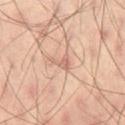| feature | finding |
|---|---|
| biopsy status | catalogued during a skin exam; not biopsied |
| body site | the right thigh |
| subject | male, aged approximately 40 |
| image | 15 mm crop, total-body photography |
| automated lesion analysis | a lesion color around L≈63 a*≈21 b*≈28 in CIELAB and a lesion-to-skin contrast of about 5.5 (normalized; higher = more distinct) |
| tile lighting | cross-polarized |